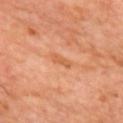Recorded during total-body skin imaging; not selected for excision or biopsy. A 15 mm close-up extracted from a 3D total-body photography capture. A male subject roughly 60 years of age. The lesion is on the upper back.